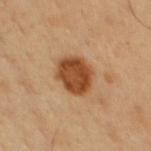Part of a total-body skin-imaging series; this lesion was reviewed on a skin check and was not flagged for biopsy.
A male subject, about 50 years old.
On the chest.
Captured under cross-polarized illumination.
A 15 mm close-up tile from a total-body photography series done for melanoma screening.
The total-body-photography lesion software estimated a mean CIELAB color near L≈47 a*≈23 b*≈38, about 15 CIELAB-L* units darker than the surrounding skin, and a lesion-to-skin contrast of about 11.5 (normalized; higher = more distinct). The software also gave border irregularity of about 1.5 on a 0–10 scale and a within-lesion color-variation index near 3.5/10.